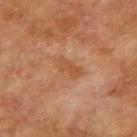<lesion>
<biopsy_status>not biopsied; imaged during a skin examination</biopsy_status>
<lighting>cross-polarized</lighting>
<lesion_size>
  <long_diameter_mm_approx>3.5</long_diameter_mm_approx>
</lesion_size>
<patient>
  <sex>male</sex>
  <age_approx>70</age_approx>
</patient>
<image>
  <source>total-body photography crop</source>
  <field_of_view_mm>15</field_of_view_mm>
</image>
<site>right upper arm</site>
</lesion>